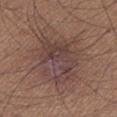{"biopsy_status": "not biopsied; imaged during a skin examination", "image": {"source": "total-body photography crop", "field_of_view_mm": 15}, "patient": {"sex": "male", "age_approx": 65}, "site": "left lower leg"}